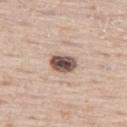  biopsy_status: not biopsied; imaged during a skin examination
  site: leg
  image:
    source: total-body photography crop
    field_of_view_mm: 15
  automated_metrics:
    area_mm2_approx: 6.5
    eccentricity: 0.7
    shape_asymmetry: 0.2
  patient:
    sex: male
    age_approx: 65
  lighting: white-light